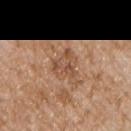No biopsy was performed on this lesion — it was imaged during a full skin examination and was not determined to be concerning. Automated tile analysis of the lesion measured a footprint of about 8 mm², a shape eccentricity near 0.65, and a shape-asymmetry score of about 0.5 (0 = symmetric). The analysis additionally found a normalized lesion–skin contrast near 6. A 15 mm close-up tile from a total-body photography series done for melanoma screening. The patient is a male roughly 65 years of age. From the left upper arm.